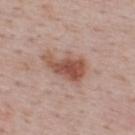Context: A male patient roughly 50 years of age. The recorded lesion diameter is about 5 mm. Captured under white-light illumination. The lesion is on the upper back. A close-up tile cropped from a whole-body skin photograph, about 15 mm across.